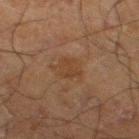{"biopsy_status": "not biopsied; imaged during a skin examination", "image": {"source": "total-body photography crop", "field_of_view_mm": 15}, "patient": {"sex": "male", "age_approx": 70}, "site": "right thigh"}